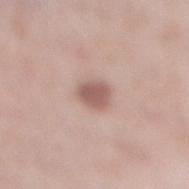biopsy_status: not biopsied; imaged during a skin examination
lighting: white-light
site: right lower leg
patient:
  sex: female
  age_approx: 40
image:
  source: total-body photography crop
  field_of_view_mm: 15